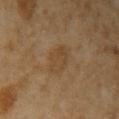acquisition: total-body-photography crop, ~15 mm field of view
body site: the arm
lighting: cross-polarized illumination
patient: female, roughly 70 years of age
automated lesion analysis: a shape eccentricity near 0.8 and a symmetry-axis asymmetry near 0.2; internal color variation of about 3 on a 0–10 scale
diameter: ≈4 mm